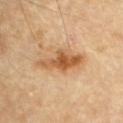Findings:
• follow-up: no biopsy performed (imaged during a skin exam)
• image-analysis metrics: an eccentricity of roughly 0.9; a within-lesion color-variation index near 6.5/10 and radial color variation of about 2; a classifier nevus-likeness of about 65/100 and lesion-presence confidence of about 100/100
• patient: male, approximately 85 years of age or older
• illumination: cross-polarized illumination
• image: ~15 mm crop, total-body skin-cancer survey
• diameter: about 6 mm
• body site: the chest